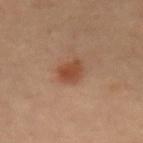The lesion was tiled from a total-body skin photograph and was not biopsied. Cropped from a whole-body photographic skin survey; the tile spans about 15 mm. The patient is a female aged 68–72. The lesion is on the front of the torso.